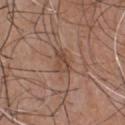site: the chest; diameter: ≈2.5 mm; TBP lesion metrics: a color-variation rating of about 4/10 and a peripheral color-asymmetry measure near 1.5; image source: 15 mm crop, total-body photography; illumination: white-light; subject: male, aged 48 to 52.